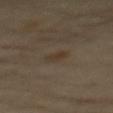follow-up = total-body-photography surveillance lesion; no biopsy | patient = male, in their 60s | body site = the mid back | acquisition = 15 mm crop, total-body photography.